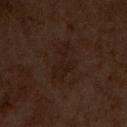follow-up — no biopsy performed (imaged during a skin exam) | automated metrics — an area of roughly 7.5 mm², an outline eccentricity of about 0.85 (0 = round, 1 = elongated), and a symmetry-axis asymmetry near 0.4; a detector confidence of about 100 out of 100 that the crop contains a lesion | patient — male, approximately 60 years of age | tile lighting — cross-polarized | diameter — about 4.5 mm | site — the front of the torso | image source — total-body-photography crop, ~15 mm field of view.